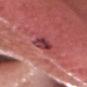The lesion was photographed on a routine skin check and not biopsied; there is no pathology result. From the head or neck. A 15 mm crop from a total-body photograph taken for skin-cancer surveillance. This is a white-light tile. A male patient, about 55 years old. The lesion-visualizer software estimated an average lesion color of about L≈40 a*≈33 b*≈20 (CIELAB) and a lesion-to-skin contrast of about 9.5 (normalized; higher = more distinct).